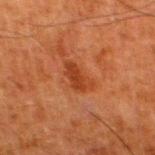| feature | finding |
|---|---|
| biopsy status | total-body-photography surveillance lesion; no biopsy |
| automated metrics | a footprint of about 6 mm², an eccentricity of roughly 0.85, and a shape-asymmetry score of about 0.35 (0 = symmetric); a border-irregularity rating of about 4/10, internal color variation of about 2 on a 0–10 scale, and radial color variation of about 0.5; lesion-presence confidence of about 100/100 |
| patient | male, aged around 80 |
| tile lighting | cross-polarized |
| acquisition | total-body-photography crop, ~15 mm field of view |
| location | the left thigh |
| size | ≈4 mm |